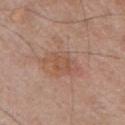Part of a total-body skin-imaging series; this lesion was reviewed on a skin check and was not flagged for biopsy. A male subject aged approximately 65. From the chest. Imaged with white-light lighting. Automated image analysis of the tile measured an average lesion color of about L≈53 a*≈21 b*≈29 (CIELAB) and a normalized lesion–skin contrast near 5.5. The software also gave a border-irregularity rating of about 4.5/10 and internal color variation of about 2.5 on a 0–10 scale. Cropped from a whole-body photographic skin survey; the tile spans about 15 mm.